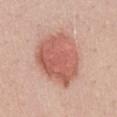{"biopsy_status": "not biopsied; imaged during a skin examination", "site": "mid back", "lighting": "white-light", "lesion_size": {"long_diameter_mm_approx": 7.0}, "image": {"source": "total-body photography crop", "field_of_view_mm": 15}, "patient": {"sex": "male", "age_approx": 40}}